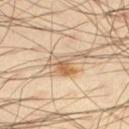workup: catalogued during a skin exam; not biopsied | imaging modality: total-body-photography crop, ~15 mm field of view | subject: male, aged around 40 | location: the left thigh.